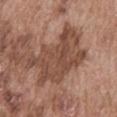No biopsy was performed on this lesion — it was imaged during a full skin examination and was not determined to be concerning. A male subject, aged 73 to 77. On the abdomen. The total-body-photography lesion software estimated a lesion area of about 27 mm², an outline eccentricity of about 0.8 (0 = round, 1 = elongated), and a shape-asymmetry score of about 0.45 (0 = symmetric). The analysis additionally found a lesion color around L≈47 a*≈20 b*≈27 in CIELAB and roughly 10 lightness units darker than nearby skin. And it measured a within-lesion color-variation index near 4/10 and peripheral color asymmetry of about 1.5. A 15 mm close-up tile from a total-body photography series done for melanoma screening.